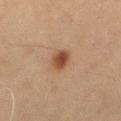* notes: imaged on a skin check; not biopsied
* site: the left thigh
* acquisition: ~15 mm crop, total-body skin-cancer survey
* tile lighting: cross-polarized
* size: about 2.5 mm
* patient: male, about 70 years old